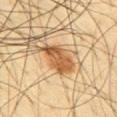Clinical summary:
The lesion is located on the mid back. The tile uses cross-polarized illumination. The recorded lesion diameter is about 5.5 mm. A male subject in their mid-40s. Cropped from a whole-body photographic skin survey; the tile spans about 15 mm.